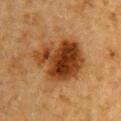The lesion was tiled from a total-body skin photograph and was not biopsied.